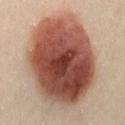• notes · total-body-photography surveillance lesion; no biopsy
• image source · ~15 mm crop, total-body skin-cancer survey
• location · the front of the torso
• illumination · cross-polarized
• diameter · ~11.5 mm (longest diameter)
• patient · female, aged 38 to 42